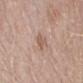<record>
  <biopsy_status>not biopsied; imaged during a skin examination</biopsy_status>
  <image>
    <source>total-body photography crop</source>
    <field_of_view_mm>15</field_of_view_mm>
  </image>
  <lighting>white-light</lighting>
  <patient>
    <sex>male</sex>
    <age_approx>50</age_approx>
  </patient>
  <site>left forearm</site>
  <lesion_size>
    <long_diameter_mm_approx>3.0</long_diameter_mm_approx>
  </lesion_size>
</record>